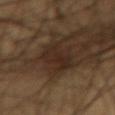| key | value |
|---|---|
| image source | ~15 mm crop, total-body skin-cancer survey |
| automated lesion analysis | a footprint of about 11 mm², an outline eccentricity of about 0.9 (0 = round, 1 = elongated), and two-axis asymmetry of about 0.3; a border-irregularity rating of about 4/10, internal color variation of about 2.5 on a 0–10 scale, and peripheral color asymmetry of about 1; a nevus-likeness score of about 20/100 and lesion-presence confidence of about 70/100 |
| location | the front of the torso |
| patient | male, aged approximately 60 |